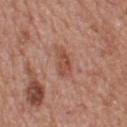Findings:
- workup — total-body-photography surveillance lesion; no biopsy
- site — the upper back
- acquisition — 15 mm crop, total-body photography
- illumination — white-light illumination
- patient — male, in their mid-70s